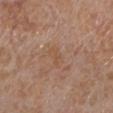Clinical impression:
Part of a total-body skin-imaging series; this lesion was reviewed on a skin check and was not flagged for biopsy.
Clinical summary:
This is a white-light tile. A female patient, in their mid- to late 50s. Longest diameter approximately 3 mm. Automated image analysis of the tile measured a mean CIELAB color near L≈52 a*≈18 b*≈31, a lesion–skin lightness drop of about 4, and a normalized lesion–skin contrast near 4.5. The software also gave a nevus-likeness score of about 0/100 and a lesion-detection confidence of about 100/100. The lesion is located on the left lower leg. A 15 mm crop from a total-body photograph taken for skin-cancer surveillance.